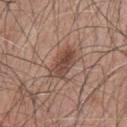notes = catalogued during a skin exam; not biopsied
size = about 4.5 mm
site = the upper back
subject = male, roughly 50 years of age
TBP lesion metrics = a lesion color around L≈46 a*≈19 b*≈25 in CIELAB, a lesion–skin lightness drop of about 11, and a normalized border contrast of about 8; a classifier nevus-likeness of about 75/100 and a lesion-detection confidence of about 100/100
image source = total-body-photography crop, ~15 mm field of view